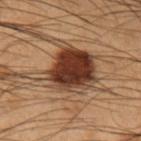Background: Cropped from a whole-body photographic skin survey; the tile spans about 15 mm. Automated tile analysis of the lesion measured a footprint of about 26 mm², an eccentricity of roughly 0.55, and a shape-asymmetry score of about 0.3 (0 = symmetric). It also reported border irregularity of about 4.5 on a 0–10 scale, a color-variation rating of about 6.5/10, and peripheral color asymmetry of about 2. And it measured a lesion-detection confidence of about 100/100. From the left forearm. About 6 mm across. The tile uses cross-polarized illumination. A male patient aged approximately 55.